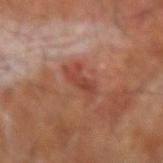Acquisition and patient details:
Cropped from a whole-body photographic skin survey; the tile spans about 15 mm. The lesion-visualizer software estimated a footprint of about 6 mm², a shape eccentricity near 0.85, and two-axis asymmetry of about 0.3. The analysis additionally found roughly 8 lightness units darker than nearby skin and a normalized border contrast of about 6.5. And it measured a lesion-detection confidence of about 100/100. Longest diameter approximately 4 mm. This is a cross-polarized tile. The lesion is located on the right forearm. A male patient aged approximately 65.